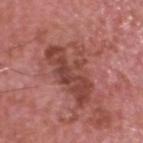biopsy_status: not biopsied; imaged during a skin examination
lighting: white-light
site: head or neck
lesion_size:
  long_diameter_mm_approx: 7.5
patient:
  sex: male
  age_approx: 75
automated_metrics:
  area_mm2_approx: 22.0
  eccentricity: 0.85
  shape_asymmetry: 0.35
  vs_skin_darker_L: 10.0
  vs_skin_contrast_norm: 7.0
  nevus_likeness_0_100: 5
image:
  source: total-body photography crop
  field_of_view_mm: 15